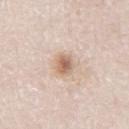{
  "biopsy_status": "not biopsied; imaged during a skin examination",
  "lighting": "white-light",
  "patient": {
    "sex": "male",
    "age_approx": 80
  },
  "image": {
    "source": "total-body photography crop",
    "field_of_view_mm": 15
  },
  "site": "leg",
  "lesion_size": {
    "long_diameter_mm_approx": 3.0
  },
  "automated_metrics": {
    "cielab_L": 65,
    "cielab_a": 17,
    "cielab_b": 29,
    "vs_skin_darker_L": 12.0,
    "vs_skin_contrast_norm": 7.5,
    "border_irregularity_0_10": 2.0,
    "color_variation_0_10": 5.0,
    "peripheral_color_asymmetry": 1.5,
    "lesion_detection_confidence_0_100": 100
  }
}